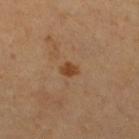follow-up = no biopsy performed (imaged during a skin exam) | subject = female, aged around 55 | automated metrics = an eccentricity of roughly 0.65; a lesion–skin lightness drop of about 9 and a normalized lesion–skin contrast near 8; border irregularity of about 2 on a 0–10 scale and a color-variation rating of about 1/10 | site = the right lower leg | size = about 2.5 mm | image source = total-body-photography crop, ~15 mm field of view | illumination = cross-polarized illumination.